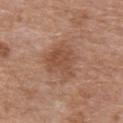Assessment:
Recorded during total-body skin imaging; not selected for excision or biopsy.
Acquisition and patient details:
A 15 mm crop from a total-body photograph taken for skin-cancer surveillance. A male subject, roughly 65 years of age. The lesion is on the chest. Automated image analysis of the tile measured a lesion area of about 14 mm² and an outline eccentricity of about 0.35 (0 = round, 1 = elongated). The analysis additionally found an average lesion color of about L≈50 a*≈21 b*≈30 (CIELAB), about 8 CIELAB-L* units darker than the surrounding skin, and a normalized lesion–skin contrast near 6.5. About 5 mm across. Imaged with white-light lighting.